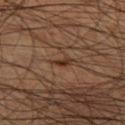  automated_metrics:
    cielab_L: 32
    cielab_a: 18
    cielab_b: 27
    vs_skin_darker_L: 8.0
    border_irregularity_0_10: 3.5
    color_variation_0_10: 0.0
    peripheral_color_asymmetry: 0.0
  site: left thigh
  lighting: cross-polarized
  image:
    source: total-body photography crop
    field_of_view_mm: 15
  lesion_size:
    long_diameter_mm_approx: 2.5
  patient:
    sex: male
    age_approx: 45